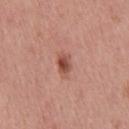Assessment: The lesion was photographed on a routine skin check and not biopsied; there is no pathology result. Background: Captured under white-light illumination. Cropped from a whole-body photographic skin survey; the tile spans about 15 mm. Automated tile analysis of the lesion measured a lesion area of about 4 mm², a shape eccentricity near 0.85, and two-axis asymmetry of about 0.2. It also reported an average lesion color of about L≈51 a*≈25 b*≈28 (CIELAB), a lesion–skin lightness drop of about 12, and a lesion-to-skin contrast of about 8.5 (normalized; higher = more distinct). The software also gave border irregularity of about 2 on a 0–10 scale, a within-lesion color-variation index near 4.5/10, and peripheral color asymmetry of about 1.5. And it measured a classifier nevus-likeness of about 95/100. The recorded lesion diameter is about 3 mm. A male subject aged 43 to 47. On the upper back.